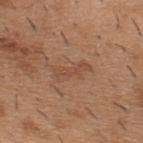workup: catalogued during a skin exam; not biopsied
subject: male, aged approximately 40
body site: the upper back
imaging modality: ~15 mm tile from a whole-body skin photo
image-analysis metrics: a mean CIELAB color near L≈48 a*≈21 b*≈31, roughly 6 lightness units darker than nearby skin, and a lesion-to-skin contrast of about 5 (normalized; higher = more distinct); border irregularity of about 5 on a 0–10 scale and a color-variation rating of about 1/10; a classifier nevus-likeness of about 0/100
diameter: about 4 mm